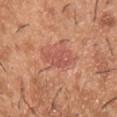Findings:
– size · ~4 mm (longest diameter)
– imaging modality · ~15 mm tile from a whole-body skin photo
– body site · the chest
– lighting · white-light
– TBP lesion metrics · an average lesion color of about L≈55 a*≈29 b*≈30 (CIELAB) and a lesion–skin lightness drop of about 8; a border-irregularity rating of about 4/10, a color-variation rating of about 2.5/10, and peripheral color asymmetry of about 1; an automated nevus-likeness rating near 25 out of 100 and lesion-presence confidence of about 100/100
– patient · male, approximately 40 years of age